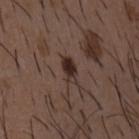biopsy status: no biopsy performed (imaged during a skin exam)
subject: male, roughly 50 years of age
image source: total-body-photography crop, ~15 mm field of view
automated lesion analysis: an average lesion color of about L≈28 a*≈15 b*≈19 (CIELAB), roughly 11 lightness units darker than nearby skin, and a normalized border contrast of about 11; a border-irregularity index near 3.5/10; a nevus-likeness score of about 90/100 and a detector confidence of about 100 out of 100 that the crop contains a lesion
lighting: white-light
lesion size: ~3.5 mm (longest diameter)
anatomic site: the chest